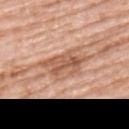Imaged during a routine full-body skin examination; the lesion was not biopsied and no histopathology is available.
Located on the left upper arm.
A female patient approximately 70 years of age.
A roughly 15 mm field-of-view crop from a total-body skin photograph.
The tile uses white-light illumination.
Measured at roughly 6 mm in maximum diameter.